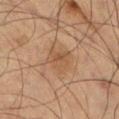Assessment:
Recorded during total-body skin imaging; not selected for excision or biopsy.
Image and clinical context:
Measured at roughly 3.5 mm in maximum diameter. A male patient aged approximately 65. From the left thigh. An algorithmic analysis of the crop reported a lesion color around L≈41 a*≈16 b*≈27 in CIELAB and a lesion–skin lightness drop of about 6. A roughly 15 mm field-of-view crop from a total-body skin photograph.